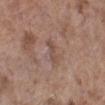No biopsy was performed on this lesion — it was imaged during a full skin examination and was not determined to be concerning.
The lesion is located on the left lower leg.
An algorithmic analysis of the crop reported a border-irregularity rating of about 3/10.
A female patient, approximately 85 years of age or older.
About 3 mm across.
Imaged with white-light lighting.
A roughly 15 mm field-of-view crop from a total-body skin photograph.